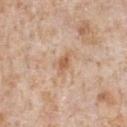This lesion was catalogued during total-body skin photography and was not selected for biopsy. The lesion's longest dimension is about 2.5 mm. From the chest. A male subject aged approximately 65. A region of skin cropped from a whole-body photographic capture, roughly 15 mm wide.